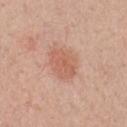Part of a total-body skin-imaging series; this lesion was reviewed on a skin check and was not flagged for biopsy.
A male patient approximately 50 years of age.
About 4 mm across.
Located on the chest.
Imaged with white-light lighting.
This image is a 15 mm lesion crop taken from a total-body photograph.